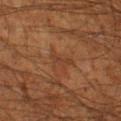| field | value |
|---|---|
| notes | catalogued during a skin exam; not biopsied |
| acquisition | 15 mm crop, total-body photography |
| site | the left thigh |
| patient | male, aged around 60 |